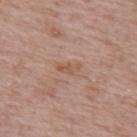Impression: Part of a total-body skin-imaging series; this lesion was reviewed on a skin check and was not flagged for biopsy. Clinical summary: The lesion is on the upper back. The subject is a female in their 60s. Captured under white-light illumination. Cropped from a whole-body photographic skin survey; the tile spans about 15 mm. The total-body-photography lesion software estimated a border-irregularity index near 4/10, internal color variation of about 0 on a 0–10 scale, and peripheral color asymmetry of about 0. The analysis additionally found a nevus-likeness score of about 0/100. Measured at roughly 2.5 mm in maximum diameter.